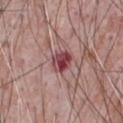Assessment: This lesion was catalogued during total-body skin photography and was not selected for biopsy. Context: The lesion is located on the chest. A roughly 15 mm field-of-view crop from a total-body skin photograph. The tile uses white-light illumination. The patient is a male aged around 70. Automated image analysis of the tile measured a lesion area of about 5.5 mm², an eccentricity of roughly 0.5, and a symmetry-axis asymmetry near 0.1. It also reported an average lesion color of about L≈43 a*≈30 b*≈19 (CIELAB) and about 14 CIELAB-L* units darker than the surrounding skin.